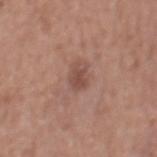biopsy_status: not biopsied; imaged during a skin examination
automated_metrics:
  area_mm2_approx: 3.5
  eccentricity: 0.8
  shape_asymmetry: 0.3
  border_irregularity_0_10: 3.5
  color_variation_0_10: 1.0
  peripheral_color_asymmetry: 0.5
  nevus_likeness_0_100: 10
site: mid back
image:
  source: total-body photography crop
  field_of_view_mm: 15
patient:
  sex: male
  age_approx: 55
lighting: white-light
lesion_size:
  long_diameter_mm_approx: 2.5